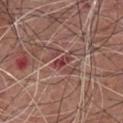* workup · no biopsy performed (imaged during a skin exam)
* image · ~15 mm crop, total-body skin-cancer survey
* body site · the front of the torso
* subject · male, aged 73 to 77